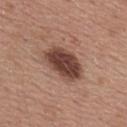Q: Was this lesion biopsied?
A: catalogued during a skin exam; not biopsied
Q: Patient demographics?
A: male, approximately 55 years of age
Q: What kind of image is this?
A: ~15 mm tile from a whole-body skin photo
Q: What is the lesion's diameter?
A: ≈5.5 mm
Q: What lighting was used for the tile?
A: white-light
Q: Lesion location?
A: the mid back